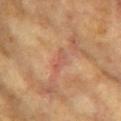The lesion was photographed on a routine skin check and not biopsied; there is no pathology result.
A 15 mm crop from a total-body photograph taken for skin-cancer surveillance.
Automated tile analysis of the lesion measured a classifier nevus-likeness of about 0/100 and lesion-presence confidence of about 85/100.
On the left upper arm.
The patient is a female aged 73 to 77.
The recorded lesion diameter is about 3 mm.